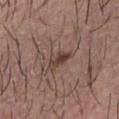Impression: Captured during whole-body skin photography for melanoma surveillance; the lesion was not biopsied. Context: The patient is a male aged around 30. The recorded lesion diameter is about 3.5 mm. A roughly 15 mm field-of-view crop from a total-body skin photograph.